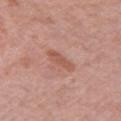Case summary:
– acquisition — ~15 mm tile from a whole-body skin photo
– TBP lesion metrics — a lesion area of about 5 mm², an outline eccentricity of about 0.9 (0 = round, 1 = elongated), and two-axis asymmetry of about 0.25; a lesion color around L≈55 a*≈24 b*≈28 in CIELAB, about 8 CIELAB-L* units darker than the surrounding skin, and a normalized lesion–skin contrast near 6; border irregularity of about 3 on a 0–10 scale and a color-variation rating of about 1.5/10; an automated nevus-likeness rating near 0 out of 100 and a lesion-detection confidence of about 100/100
– subject — female, approximately 60 years of age
– size — ~4 mm (longest diameter)
– illumination — white-light illumination
– body site — the left upper arm